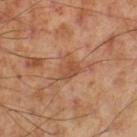Captured during whole-body skin photography for melanoma surveillance; the lesion was not biopsied. The tile uses cross-polarized illumination. A male subject aged 53 to 57. On the left thigh. About 3 mm across. A roughly 15 mm field-of-view crop from a total-body skin photograph.